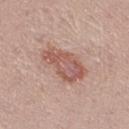Findings:
– lesion size · about 6 mm
– subject · female, aged 23–27
– acquisition · ~15 mm tile from a whole-body skin photo
– site · the leg